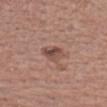Q: Is there a histopathology result?
A: total-body-photography surveillance lesion; no biopsy
Q: How was the tile lit?
A: white-light illumination
Q: What is the imaging modality?
A: total-body-photography crop, ~15 mm field of view
Q: What is the anatomic site?
A: the front of the torso
Q: What is the lesion's diameter?
A: about 3 mm
Q: Who is the patient?
A: male, in their 70s